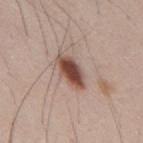The lesion was photographed on a routine skin check and not biopsied; there is no pathology result. On the mid back. Automated image analysis of the tile measured an average lesion color of about L≈48 a*≈20 b*≈25 (CIELAB) and about 17 CIELAB-L* units darker than the surrounding skin. It also reported lesion-presence confidence of about 100/100. A male patient, approximately 35 years of age. Cropped from a total-body skin-imaging series; the visible field is about 15 mm.